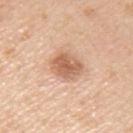imaging modality: ~15 mm tile from a whole-body skin photo
location: the left upper arm
lesion size: ~3.5 mm (longest diameter)
image-analysis metrics: a footprint of about 8.5 mm², an eccentricity of roughly 0.45, and a shape-asymmetry score of about 0.1 (0 = symmetric); a mean CIELAB color near L≈62 a*≈22 b*≈33, a lesion–skin lightness drop of about 13, and a lesion-to-skin contrast of about 8 (normalized; higher = more distinct); a classifier nevus-likeness of about 65/100 and lesion-presence confidence of about 100/100
tile lighting: white-light illumination
patient: male, approximately 35 years of age